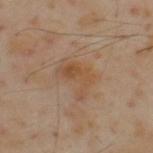The lesion was tiled from a total-body skin photograph and was not biopsied. The lesion is located on the upper back. A 15 mm close-up extracted from a 3D total-body photography capture. The patient is a male aged around 55.